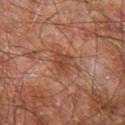  biopsy_status: not biopsied; imaged during a skin examination
  image:
    source: total-body photography crop
    field_of_view_mm: 15
  automated_metrics:
    cielab_L: 42
    cielab_a: 24
    cielab_b: 30
    vs_skin_darker_L: 8.0
    vs_skin_contrast_norm: 6.5
    nevus_likeness_0_100: 30
    lesion_detection_confidence_0_100: 100
  lesion_size:
    long_diameter_mm_approx: 3.0
  lighting: cross-polarized
  site: leg
  patient:
    sex: male
    age_approx: 60